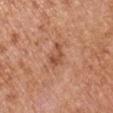Impression:
Captured during whole-body skin photography for melanoma surveillance; the lesion was not biopsied.
Context:
The lesion's longest dimension is about 3 mm. A male patient, in their mid-60s. Cropped from a total-body skin-imaging series; the visible field is about 15 mm. The tile uses white-light illumination. The lesion-visualizer software estimated roughly 10 lightness units darker than nearby skin and a normalized lesion–skin contrast near 7. The software also gave an automated nevus-likeness rating near 15 out of 100 and a lesion-detection confidence of about 100/100. The lesion is located on the arm.